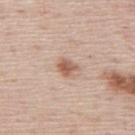Recorded during total-body skin imaging; not selected for excision or biopsy. This is a white-light tile. From the mid back. The lesion-visualizer software estimated a shape eccentricity near 0.6 and a shape-asymmetry score of about 0.25 (0 = symmetric). And it measured an average lesion color of about L≈59 a*≈21 b*≈29 (CIELAB) and a normalized border contrast of about 8. The software also gave a border-irregularity rating of about 2.5/10, a within-lesion color-variation index near 3/10, and peripheral color asymmetry of about 1. Measured at roughly 2.5 mm in maximum diameter. A male patient aged 43 to 47. A roughly 15 mm field-of-view crop from a total-body skin photograph.